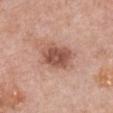Notes:
* biopsy status · no biopsy performed (imaged during a skin exam)
* automated metrics · an area of roughly 12 mm², a shape eccentricity near 0.7, and two-axis asymmetry of about 0.15; about 13 CIELAB-L* units darker than the surrounding skin; border irregularity of about 2 on a 0–10 scale, a color-variation rating of about 4/10, and peripheral color asymmetry of about 1.5; a nevus-likeness score of about 50/100 and a detector confidence of about 100 out of 100 that the crop contains a lesion
* image source · ~15 mm crop, total-body skin-cancer survey
* patient · female, approximately 60 years of age
* size · about 4.5 mm
* site · the chest
* tile lighting · white-light